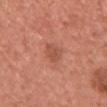No biopsy was performed on this lesion — it was imaged during a full skin examination and was not determined to be concerning.
The lesion is located on the chest.
A 15 mm close-up tile from a total-body photography series done for melanoma screening.
The subject is a female aged around 40.